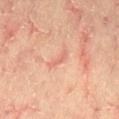lesion diameter: ~3 mm (longest diameter) | acquisition: ~15 mm crop, total-body skin-cancer survey | lighting: cross-polarized | site: the lower back | subject: male, roughly 65 years of age.